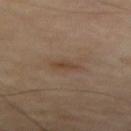site = the right thigh | imaging modality = 15 mm crop, total-body photography | patient = male, aged 68 to 72 | size = about 2.5 mm | automated lesion analysis = border irregularity of about 2.5 on a 0–10 scale, a color-variation rating of about 2/10, and a peripheral color-asymmetry measure near 0.5; a classifier nevus-likeness of about 5/100.